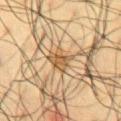Clinical summary: About 4 mm across. Automated image analysis of the tile measured a footprint of about 6.5 mm², an eccentricity of roughly 0.75, and a symmetry-axis asymmetry near 0.3. And it measured a lesion color around L≈44 a*≈13 b*≈31 in CIELAB, a lesion–skin lightness drop of about 9, and a normalized lesion–skin contrast near 7.5. The analysis additionally found a border-irregularity index near 3.5/10 and internal color variation of about 2.5 on a 0–10 scale. On the chest. The subject is a male about 60 years old. This is a cross-polarized tile. A close-up tile cropped from a whole-body skin photograph, about 15 mm across.